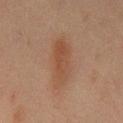Longest diameter approximately 7 mm. Imaged with cross-polarized lighting. The subject is a male aged around 45. A 15 mm crop from a total-body photograph taken for skin-cancer surveillance. The lesion is located on the chest.